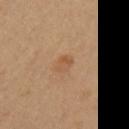Part of a total-body skin-imaging series; this lesion was reviewed on a skin check and was not flagged for biopsy. Captured under cross-polarized illumination. The patient is a female roughly 40 years of age. On the left upper arm. Automated tile analysis of the lesion measured an area of roughly 3 mm² and an outline eccentricity of about 0.8 (0 = round, 1 = elongated). And it measured a lesion color around L≈54 a*≈20 b*≈35 in CIELAB. The software also gave border irregularity of about 2 on a 0–10 scale. Measured at roughly 2.5 mm in maximum diameter. This image is a 15 mm lesion crop taken from a total-body photograph.